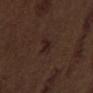Assessment: Recorded during total-body skin imaging; not selected for excision or biopsy. Context: A male patient approximately 70 years of age. The lesion is located on the back. A roughly 15 mm field-of-view crop from a total-body skin photograph. The tile uses white-light illumination. Automated tile analysis of the lesion measured an area of roughly 5.5 mm² and a shape eccentricity near 0.75. It also reported a border-irregularity index near 3/10, internal color variation of about 3 on a 0–10 scale, and a peripheral color-asymmetry measure near 1. Longest diameter approximately 3 mm.